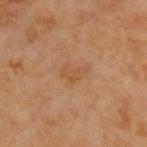{"biopsy_status": "not biopsied; imaged during a skin examination", "lesion_size": {"long_diameter_mm_approx": 3.0}, "site": "chest", "image": {"source": "total-body photography crop", "field_of_view_mm": 15}, "patient": {"sex": "male", "age_approx": 65}}